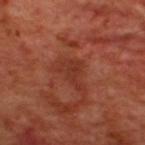The lesion was tiled from a total-body skin photograph and was not biopsied.
A 15 mm close-up tile from a total-body photography series done for melanoma screening.
From the upper back.
Approximately 3.5 mm at its widest.
A male patient, roughly 50 years of age.
The tile uses cross-polarized illumination.